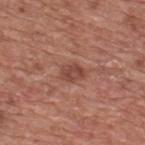Captured under white-light illumination.
The lesion is on the upper back.
The lesion-visualizer software estimated a mean CIELAB color near L≈45 a*≈25 b*≈27, roughly 9 lightness units darker than nearby skin, and a normalized lesion–skin contrast near 7. The software also gave border irregularity of about 2 on a 0–10 scale and a within-lesion color-variation index near 2.5/10.
This image is a 15 mm lesion crop taken from a total-body photograph.
A male subject about 75 years old.